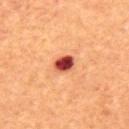Image and clinical context: A region of skin cropped from a whole-body photographic capture, roughly 15 mm wide. From the front of the torso. The patient is a male about 65 years old. Automated tile analysis of the lesion measured an area of roughly 5 mm² and a shape eccentricity near 0.65. And it measured a mean CIELAB color near L≈46 a*≈34 b*≈32 and roughly 21 lightness units darker than nearby skin. The analysis additionally found a border-irregularity rating of about 1.5/10 and a peripheral color-asymmetry measure near 2. And it measured a classifier nevus-likeness of about 0/100 and a detector confidence of about 100 out of 100 that the crop contains a lesion. Imaged with cross-polarized lighting.